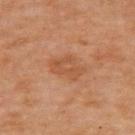Captured during whole-body skin photography for melanoma surveillance; the lesion was not biopsied.